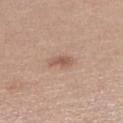Assessment: Imaged during a routine full-body skin examination; the lesion was not biopsied and no histopathology is available. Image and clinical context: A roughly 15 mm field-of-view crop from a total-body skin photograph. About 2.5 mm across. Captured under white-light illumination. Located on the right lower leg. The patient is a female in their mid-60s.